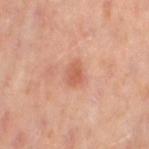workup=catalogued during a skin exam; not biopsied